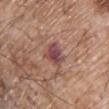Q: What are the patient's age and sex?
A: male, aged approximately 65
Q: How large is the lesion?
A: ~3 mm (longest diameter)
Q: How was the tile lit?
A: white-light illumination
Q: How was this image acquired?
A: total-body-photography crop, ~15 mm field of view
Q: What is the anatomic site?
A: the chest
Q: What did automated image analysis measure?
A: a border-irregularity rating of about 2.5/10, a color-variation rating of about 4.5/10, and radial color variation of about 1.5